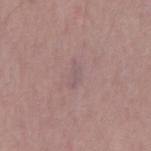The lesion was tiled from a total-body skin photograph and was not biopsied.
A male subject, in their mid-50s.
Cropped from a whole-body photographic skin survey; the tile spans about 15 mm.
Automated tile analysis of the lesion measured a lesion area of about 3 mm² and an outline eccentricity of about 0.9 (0 = round, 1 = elongated). The software also gave an average lesion color of about L≈54 a*≈17 b*≈15 (CIELAB), roughly 5 lightness units darker than nearby skin, and a normalized lesion–skin contrast near 4.5. The analysis additionally found a classifier nevus-likeness of about 0/100 and lesion-presence confidence of about 55/100.
Longest diameter approximately 3 mm.